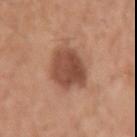Case summary:
• workup: total-body-photography surveillance lesion; no biopsy
• image-analysis metrics: about 13 CIELAB-L* units darker than the surrounding skin and a lesion-to-skin contrast of about 9 (normalized; higher = more distinct); border irregularity of about 2.5 on a 0–10 scale, a within-lesion color-variation index near 4/10, and a peripheral color-asymmetry measure near 1.5
• imaging modality: ~15 mm tile from a whole-body skin photo
• subject: male, roughly 55 years of age
• location: the left upper arm
• illumination: white-light